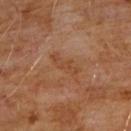No biopsy was performed on this lesion — it was imaged during a full skin examination and was not determined to be concerning. Measured at roughly 4 mm in maximum diameter. Imaged with cross-polarized lighting. A 15 mm close-up extracted from a 3D total-body photography capture. The patient is a male roughly 60 years of age. Located on the chest.